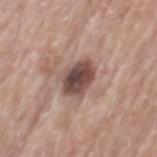– workup · catalogued during a skin exam; not biopsied
– image source · ~15 mm tile from a whole-body skin photo
– body site · the mid back
– patient · male, aged approximately 70
– lighting · white-light illumination